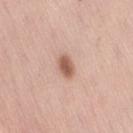The lesion was tiled from a total-body skin photograph and was not biopsied. On the lower back. The subject is a female aged around 60. Measured at roughly 3 mm in maximum diameter. This is a white-light tile. A 15 mm close-up extracted from a 3D total-body photography capture. Automated image analysis of the tile measured an outline eccentricity of about 0.75 (0 = round, 1 = elongated) and a shape-asymmetry score of about 0.25 (0 = symmetric). The analysis additionally found roughly 14 lightness units darker than nearby skin and a lesion-to-skin contrast of about 9 (normalized; higher = more distinct). And it measured a within-lesion color-variation index near 1.5/10. The software also gave lesion-presence confidence of about 100/100.